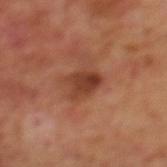Clinical impression: Part of a total-body skin-imaging series; this lesion was reviewed on a skin check and was not flagged for biopsy. Context: From the mid back. A male patient, aged 68 to 72. Measured at roughly 3.5 mm in maximum diameter. A lesion tile, about 15 mm wide, cut from a 3D total-body photograph.